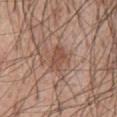Part of a total-body skin-imaging series; this lesion was reviewed on a skin check and was not flagged for biopsy.